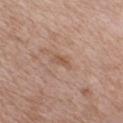Impression:
This lesion was catalogued during total-body skin photography and was not selected for biopsy.
Background:
On the chest. A male subject approximately 50 years of age. A close-up tile cropped from a whole-body skin photograph, about 15 mm across.